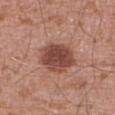biopsy status — total-body-photography surveillance lesion; no biopsy | image — total-body-photography crop, ~15 mm field of view | anatomic site — the abdomen | size — about 4.5 mm | patient — male, aged approximately 75.